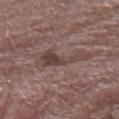Impression: The lesion was photographed on a routine skin check and not biopsied; there is no pathology result. Image and clinical context: The patient is a male aged 73 to 77. The recorded lesion diameter is about 6 mm. A roughly 15 mm field-of-view crop from a total-body skin photograph. This is a white-light tile. From the left forearm.